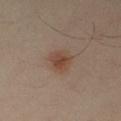{
  "biopsy_status": "not biopsied; imaged during a skin examination",
  "lesion_size": {
    "long_diameter_mm_approx": 3.0
  },
  "site": "arm",
  "patient": {
    "sex": "male",
    "age_approx": 40
  },
  "image": {
    "source": "total-body photography crop",
    "field_of_view_mm": 15
  }
}